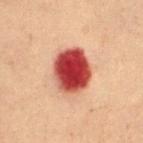Assessment:
Captured during whole-body skin photography for melanoma surveillance; the lesion was not biopsied.
Background:
A roughly 15 mm field-of-view crop from a total-body skin photograph. An algorithmic analysis of the crop reported a nevus-likeness score of about 0/100. Located on the mid back. Captured under cross-polarized illumination. The lesion's longest dimension is about 5 mm. The subject is a female in their 80s.